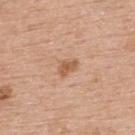Q: Was this lesion biopsied?
A: no biopsy performed (imaged during a skin exam)
Q: Lesion size?
A: ~2.5 mm (longest diameter)
Q: How was this image acquired?
A: total-body-photography crop, ~15 mm field of view
Q: What lighting was used for the tile?
A: white-light
Q: Lesion location?
A: the upper back
Q: Who is the patient?
A: female, aged around 65